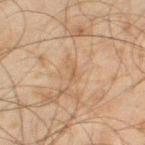Q: Was this lesion biopsied?
A: catalogued during a skin exam; not biopsied
Q: Lesion location?
A: the left thigh
Q: What kind of image is this?
A: ~15 mm crop, total-body skin-cancer survey
Q: Patient demographics?
A: male, roughly 45 years of age
Q: Lesion size?
A: ~3 mm (longest diameter)